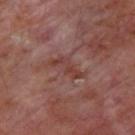Impression: Captured during whole-body skin photography for melanoma surveillance; the lesion was not biopsied. Image and clinical context: This is a cross-polarized tile. On the upper back. Cropped from a total-body skin-imaging series; the visible field is about 15 mm. A subject about 55 years old. Longest diameter approximately 4 mm.